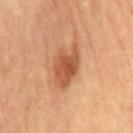<record>
<biopsy_status>not biopsied; imaged during a skin examination</biopsy_status>
<lesion_size>
  <long_diameter_mm_approx>5.5</long_diameter_mm_approx>
</lesion_size>
<site>chest</site>
<lighting>cross-polarized</lighting>
<automated_metrics>
  <cielab_L>54</cielab_L>
  <cielab_a>25</cielab_a>
  <cielab_b>36</cielab_b>
  <vs_skin_darker_L>12.0</vs_skin_darker_L>
  <vs_skin_contrast_norm>8.0</vs_skin_contrast_norm>
  <border_irregularity_0_10>3.5</border_irregularity_0_10>
  <color_variation_0_10>4.0</color_variation_0_10>
  <peripheral_color_asymmetry>1.0</peripheral_color_asymmetry>
</automated_metrics>
<image>
  <source>total-body photography crop</source>
  <field_of_view_mm>15</field_of_view_mm>
</image>
<patient>
  <sex>male</sex>
  <age_approx>85+</age_approx>
</patient>
</record>